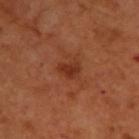{
  "biopsy_status": "not biopsied; imaged during a skin examination",
  "lighting": "cross-polarized",
  "patient": {
    "sex": "male",
    "age_approx": 50
  },
  "lesion_size": {
    "long_diameter_mm_approx": 2.5
  },
  "image": {
    "source": "total-body photography crop",
    "field_of_view_mm": 15
  },
  "site": "upper back"
}